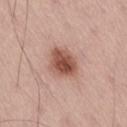<case>
  <biopsy_status>not biopsied; imaged during a skin examination</biopsy_status>
  <lesion_size>
    <long_diameter_mm_approx>3.5</long_diameter_mm_approx>
  </lesion_size>
  <patient>
    <sex>male</sex>
    <age_approx>55</age_approx>
  </patient>
  <image>
    <source>total-body photography crop</source>
    <field_of_view_mm>15</field_of_view_mm>
  </image>
  <automated_metrics>
    <area_mm2_approx>10.0</area_mm2_approx>
    <shape_asymmetry>0.2</shape_asymmetry>
    <cielab_L>52</cielab_L>
    <cielab_a>23</cielab_a>
    <cielab_b>28</cielab_b>
    <vs_skin_darker_L>15.0</vs_skin_darker_L>
    <vs_skin_contrast_norm>10.0</vs_skin_contrast_norm>
    <border_irregularity_0_10>2.0</border_irregularity_0_10>
    <color_variation_0_10>5.5</color_variation_0_10>
    <peripheral_color_asymmetry>2.0</peripheral_color_asymmetry>
    <nevus_likeness_0_100>95</nevus_likeness_0_100>
    <lesion_detection_confidence_0_100>100</lesion_detection_confidence_0_100>
  </automated_metrics>
  <site>right thigh</site>
</case>